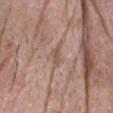Q: Was a biopsy performed?
A: catalogued during a skin exam; not biopsied
Q: How was the tile lit?
A: white-light
Q: What is the lesion's diameter?
A: about 3 mm
Q: Where on the body is the lesion?
A: the head or neck
Q: How was this image acquired?
A: 15 mm crop, total-body photography
Q: What are the patient's age and sex?
A: male, aged approximately 75